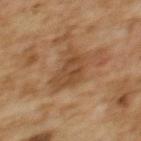{
  "biopsy_status": "not biopsied; imaged during a skin examination",
  "lesion_size": {
    "long_diameter_mm_approx": 4.5
  },
  "lighting": "cross-polarized",
  "image": {
    "source": "total-body photography crop",
    "field_of_view_mm": 15
  },
  "site": "upper back",
  "patient": {
    "sex": "female",
    "age_approx": 60
  },
  "automated_metrics": {
    "area_mm2_approx": 12.0,
    "eccentricity": 0.5,
    "shape_asymmetry": 0.35,
    "cielab_L": 40,
    "cielab_a": 17,
    "cielab_b": 31,
    "vs_skin_darker_L": 7.0,
    "vs_skin_contrast_norm": 6.5,
    "peripheral_color_asymmetry": 1.0,
    "nevus_likeness_0_100": 0,
    "lesion_detection_confidence_0_100": 100
  }
}